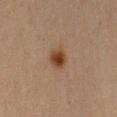<tbp_lesion>
<biopsy_status>not biopsied; imaged during a skin examination</biopsy_status>
<image>
  <source>total-body photography crop</source>
  <field_of_view_mm>15</field_of_view_mm>
</image>
<lighting>cross-polarized</lighting>
<site>left upper arm</site>
<patient>
  <sex>female</sex>
  <age_approx>40</age_approx>
</patient>
<lesion_size>
  <long_diameter_mm_approx>2.5</long_diameter_mm_approx>
</lesion_size>
</tbp_lesion>